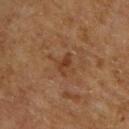  lighting: cross-polarized
  site: upper back
  patient:
    sex: male
    age_approx: 65
  image:
    source: total-body photography crop
    field_of_view_mm: 15
  automated_metrics:
    vs_skin_darker_L: 7.0
    vs_skin_contrast_norm: 6.5
    nevus_likeness_0_100: 0
  lesion_size:
    long_diameter_mm_approx: 2.5